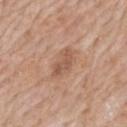Impression: Captured during whole-body skin photography for melanoma surveillance; the lesion was not biopsied. Background: The lesion's longest dimension is about 3.5 mm. The lesion is on the mid back. A 15 mm close-up extracted from a 3D total-body photography capture. The total-body-photography lesion software estimated an area of roughly 5 mm² and an outline eccentricity of about 0.8 (0 = round, 1 = elongated). The patient is a male aged around 60.